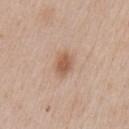<record>
  <biopsy_status>not biopsied; imaged during a skin examination</biopsy_status>
  <patient>
    <sex>male</sex>
    <age_approx>60</age_approx>
  </patient>
  <site>chest</site>
  <image>
    <source>total-body photography crop</source>
    <field_of_view_mm>15</field_of_view_mm>
  </image>
</record>